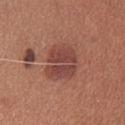biopsy status: catalogued during a skin exam; not biopsied | automated metrics: a footprint of about 12 mm² and a shape-asymmetry score of about 0.25 (0 = symmetric); a border-irregularity index near 6/10, internal color variation of about 3 on a 0–10 scale, and a peripheral color-asymmetry measure near 1; an automated nevus-likeness rating near 90 out of 100 and lesion-presence confidence of about 100/100 | image: ~15 mm tile from a whole-body skin photo | diameter: ≈4 mm | lighting: white-light illumination | anatomic site: the head or neck | patient: female, approximately 35 years of age.